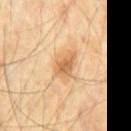| field | value |
|---|---|
| anatomic site | the chest |
| TBP lesion metrics | a shape eccentricity near 0.75 and a shape-asymmetry score of about 0.3 (0 = symmetric); border irregularity of about 3 on a 0–10 scale, a within-lesion color-variation index near 4/10, and a peripheral color-asymmetry measure near 1 |
| patient | male, aged 68 to 72 |
| tile lighting | cross-polarized |
| acquisition | ~15 mm crop, total-body skin-cancer survey |
| lesion size | about 3.5 mm |